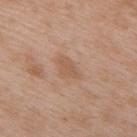Captured during whole-body skin photography for melanoma surveillance; the lesion was not biopsied.